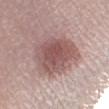Case summary:
– automated lesion analysis: a lesion area of about 27 mm² and a shape-asymmetry score of about 0.2 (0 = symmetric); an automated nevus-likeness rating near 75 out of 100 and lesion-presence confidence of about 100/100
– patient: female, in their 30s
– lesion size: about 6 mm
– acquisition: ~15 mm crop, total-body skin-cancer survey
– anatomic site: the left lower leg